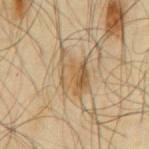biopsy status: imaged on a skin check; not biopsied
acquisition: ~15 mm crop, total-body skin-cancer survey
body site: the mid back
patient: male, approximately 65 years of age